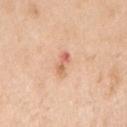{"biopsy_status": "not biopsied; imaged during a skin examination", "patient": {"sex": "male", "age_approx": 70}, "site": "right upper arm", "image": {"source": "total-body photography crop", "field_of_view_mm": 15}}